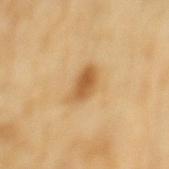workup: total-body-photography surveillance lesion; no biopsy | diameter: ≈3.5 mm | body site: the mid back | patient: female, aged approximately 70 | image: ~15 mm tile from a whole-body skin photo.